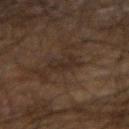Imaged during a routine full-body skin examination; the lesion was not biopsied and no histopathology is available. A male patient roughly 70 years of age. The lesion is on the right forearm. A roughly 15 mm field-of-view crop from a total-body skin photograph.